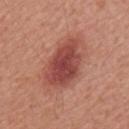  lighting: white-light
  image:
    source: total-body photography crop
    field_of_view_mm: 15
  site: mid back
  patient:
    sex: male
    age_approx: 65
  automated_metrics:
    cielab_L: 47
    cielab_a: 29
    cielab_b: 26
    vs_skin_darker_L: 13.0
    border_irregularity_0_10: 2.5
    color_variation_0_10: 4.0
    peripheral_color_asymmetry: 1.0
  lesion_size:
    long_diameter_mm_approx: 7.0